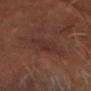No biopsy was performed on this lesion — it was imaged during a full skin examination and was not determined to be concerning.
The lesion-visualizer software estimated a shape eccentricity near 0.9. It also reported a lesion color around L≈30 a*≈18 b*≈21 in CIELAB, roughly 4 lightness units darker than nearby skin, and a normalized lesion–skin contrast near 5.
A close-up tile cropped from a whole-body skin photograph, about 15 mm across.
A male patient, in their mid- to late 60s.
Approximately 6 mm at its widest.
Located on the head or neck.
The tile uses cross-polarized illumination.